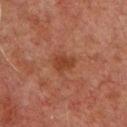The lesion was photographed on a routine skin check and not biopsied; there is no pathology result.